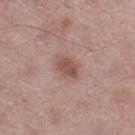Clinical impression: This lesion was catalogued during total-body skin photography and was not selected for biopsy. Image and clinical context: A male patient, about 55 years old. Measured at roughly 3 mm in maximum diameter. Automated tile analysis of the lesion measured a footprint of about 5 mm², an eccentricity of roughly 0.65, and two-axis asymmetry of about 0.15. The software also gave a border-irregularity rating of about 1.5/10, internal color variation of about 2 on a 0–10 scale, and a peripheral color-asymmetry measure near 0.5. The analysis additionally found an automated nevus-likeness rating near 70 out of 100 and a detector confidence of about 100 out of 100 that the crop contains a lesion. The lesion is on the right thigh. Cropped from a whole-body photographic skin survey; the tile spans about 15 mm.